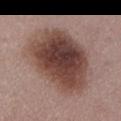The total-body-photography lesion software estimated a lesion area of about 46 mm² and a symmetry-axis asymmetry near 0.2. The analysis additionally found a border-irregularity index near 2/10, internal color variation of about 7 on a 0–10 scale, and a peripheral color-asymmetry measure near 2. The software also gave an automated nevus-likeness rating near 80 out of 100 and a detector confidence of about 100 out of 100 that the crop contains a lesion.
About 9 mm across.
A 15 mm close-up tile from a total-body photography series done for melanoma screening.
Located on the abdomen.
Imaged with white-light lighting.
The subject is a female aged approximately 50.